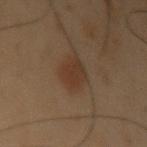Q: Was this lesion biopsied?
A: imaged on a skin check; not biopsied
Q: How large is the lesion?
A: about 3.5 mm
Q: Illumination type?
A: cross-polarized
Q: Who is the patient?
A: male, aged 53 to 57
Q: What kind of image is this?
A: 15 mm crop, total-body photography
Q: Where on the body is the lesion?
A: the left upper arm